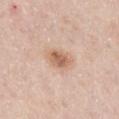{
  "automated_metrics": {
    "area_mm2_approx": 7.0,
    "eccentricity": 0.8,
    "shape_asymmetry": 0.2,
    "cielab_L": 63,
    "cielab_a": 19,
    "cielab_b": 31,
    "vs_skin_darker_L": 11.0,
    "vs_skin_contrast_norm": 7.5,
    "nevus_likeness_0_100": 75,
    "lesion_detection_confidence_0_100": 100
  },
  "patient": {
    "sex": "male",
    "age_approx": 40
  },
  "site": "mid back",
  "lesion_size": {
    "long_diameter_mm_approx": 4.0
  },
  "image": {
    "source": "total-body photography crop",
    "field_of_view_mm": 15
  },
  "lighting": "white-light"
}